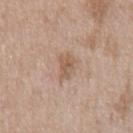The lesion was photographed on a routine skin check and not biopsied; there is no pathology result. Imaged with white-light lighting. Cropped from a whole-body photographic skin survey; the tile spans about 15 mm. On the front of the torso. Automated tile analysis of the lesion measured an area of roughly 4.5 mm² and a symmetry-axis asymmetry near 0.25. The analysis additionally found an automated nevus-likeness rating near 0 out of 100. The subject is a male aged around 50.